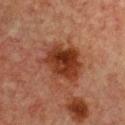{
  "biopsy_status": "not biopsied; imaged during a skin examination",
  "image": {
    "source": "total-body photography crop",
    "field_of_view_mm": 15
  },
  "lighting": "cross-polarized",
  "patient": {
    "sex": "female",
    "age_approx": 55
  },
  "site": "chest"
}